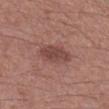{
  "biopsy_status": "not biopsied; imaged during a skin examination",
  "site": "left forearm",
  "image": {
    "source": "total-body photography crop",
    "field_of_view_mm": 15
  },
  "patient": {
    "sex": "female",
    "age_approx": 55
  }
}